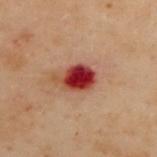{
  "biopsy_status": "not biopsied; imaged during a skin examination",
  "image": {
    "source": "total-body photography crop",
    "field_of_view_mm": 15
  },
  "lesion_size": {
    "long_diameter_mm_approx": 4.0
  },
  "automated_metrics": {
    "area_mm2_approx": 8.0,
    "eccentricity": 0.7,
    "cielab_L": 34,
    "cielab_a": 33,
    "cielab_b": 27,
    "vs_skin_darker_L": 18.0,
    "vs_skin_contrast_norm": 14.5,
    "nevus_likeness_0_100": 0,
    "lesion_detection_confidence_0_100": 100
  },
  "patient": {
    "sex": "female",
    "age_approx": 60
  },
  "site": "upper back",
  "lighting": "cross-polarized"
}